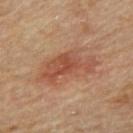The lesion was photographed on a routine skin check and not biopsied; there is no pathology result.